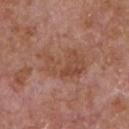biopsy status: imaged on a skin check; not biopsied | body site: the chest | automated lesion analysis: a footprint of about 17 mm², an outline eccentricity of about 0.8 (0 = round, 1 = elongated), and a shape-asymmetry score of about 0.4 (0 = symmetric); a mean CIELAB color near L≈48 a*≈22 b*≈30, about 6 CIELAB-L* units darker than the surrounding skin, and a lesion-to-skin contrast of about 6 (normalized; higher = more distinct); a border-irregularity index near 6/10 and a within-lesion color-variation index near 4/10; an automated nevus-likeness rating near 0 out of 100 | illumination: white-light | imaging modality: 15 mm crop, total-body photography | subject: male, approximately 65 years of age | size: ~6.5 mm (longest diameter).